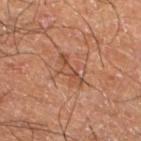Clinical impression: Captured during whole-body skin photography for melanoma surveillance; the lesion was not biopsied. Image and clinical context: A 15 mm close-up tile from a total-body photography series done for melanoma screening. Longest diameter approximately 4 mm. The total-body-photography lesion software estimated a footprint of about 4.5 mm², an outline eccentricity of about 0.95 (0 = round, 1 = elongated), and two-axis asymmetry of about 0.45. The software also gave roughly 8 lightness units darker than nearby skin. And it measured a nevus-likeness score of about 0/100 and lesion-presence confidence of about 95/100. The lesion is on the left lower leg. A male patient, aged around 60.